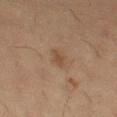Image and clinical context: The lesion's longest dimension is about 2.5 mm. Cropped from a total-body skin-imaging series; the visible field is about 15 mm. Imaged with cross-polarized lighting. A female subject about 55 years old. Located on the left thigh.